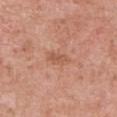<tbp_lesion>
<biopsy_status>not biopsied; imaged during a skin examination</biopsy_status>
</tbp_lesion>